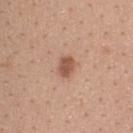Q: Was a biopsy performed?
A: total-body-photography surveillance lesion; no biopsy
Q: Illumination type?
A: white-light
Q: What kind of image is this?
A: 15 mm crop, total-body photography
Q: Where on the body is the lesion?
A: the upper back
Q: Patient demographics?
A: female, about 40 years old
Q: Lesion size?
A: about 2.5 mm